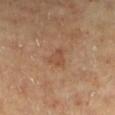{
  "biopsy_status": "not biopsied; imaged during a skin examination",
  "lesion_size": {
    "long_diameter_mm_approx": 2.5
  },
  "automated_metrics": {
    "border_irregularity_0_10": 4.5,
    "color_variation_0_10": 1.5,
    "peripheral_color_asymmetry": 0.5
  },
  "patient": {
    "sex": "female",
    "age_approx": 60
  },
  "site": "left lower leg",
  "image": {
    "source": "total-body photography crop",
    "field_of_view_mm": 15
  },
  "lighting": "cross-polarized"
}